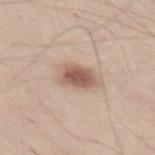<lesion>
  <biopsy_status>not biopsied; imaged during a skin examination</biopsy_status>
  <lighting>white-light</lighting>
  <lesion_size>
    <long_diameter_mm_approx>4.0</long_diameter_mm_approx>
  </lesion_size>
  <patient>
    <sex>male</sex>
    <age_approx>45</age_approx>
  </patient>
  <site>left thigh</site>
  <image>
    <source>total-body photography crop</source>
    <field_of_view_mm>15</field_of_view_mm>
  </image>
</lesion>